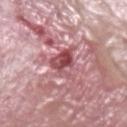lighting: white-light
patient:
  sex: male
  age_approx: 40
image:
  source: total-body photography crop
  field_of_view_mm: 15
site: right lower leg
lesion_size:
  long_diameter_mm_approx: 4.5